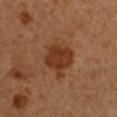Captured during whole-body skin photography for melanoma surveillance; the lesion was not biopsied.
The subject is a male in their 50s.
This image is a 15 mm lesion crop taken from a total-body photograph.
From the left upper arm.